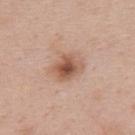workup: total-body-photography surveillance lesion; no biopsy | subject: female, roughly 35 years of age | location: the upper back | TBP lesion metrics: an area of roughly 7.5 mm², a shape eccentricity near 0.6, and a shape-asymmetry score of about 0.2 (0 = symmetric); a nevus-likeness score of about 85/100 and lesion-presence confidence of about 100/100 | acquisition: ~15 mm crop, total-body skin-cancer survey | tile lighting: white-light.